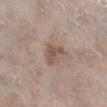Image and clinical context:
A female subject, about 75 years old. Longest diameter approximately 3.5 mm. A region of skin cropped from a whole-body photographic capture, roughly 15 mm wide. Captured under white-light illumination. The lesion is on the right lower leg.